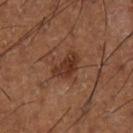image: ~15 mm crop, total-body skin-cancer survey | body site: the left lower leg | patient: male, aged 63–67 | tile lighting: cross-polarized illumination | TBP lesion metrics: a footprint of about 6.5 mm² and an eccentricity of roughly 0.8; a nevus-likeness score of about 75/100 and a lesion-detection confidence of about 100/100.